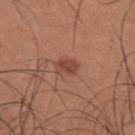Findings:
- follow-up — total-body-photography surveillance lesion; no biopsy
- body site — the upper back
- lighting — white-light illumination
- patient — male, aged 33–37
- image source — 15 mm crop, total-body photography